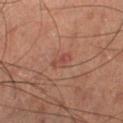Case summary:
– follow-up · no biopsy performed (imaged during a skin exam)
– subject · male, aged around 60
– body site · the left lower leg
– imaging modality · ~15 mm crop, total-body skin-cancer survey
– lighting · cross-polarized illumination